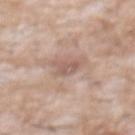Recorded during total-body skin imaging; not selected for excision or biopsy. A 15 mm close-up extracted from a 3D total-body photography capture. This is a white-light tile. The lesion is located on the front of the torso. A male subject roughly 60 years of age.